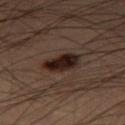notes: imaged on a skin check; not biopsied
anatomic site: the right thigh
acquisition: ~15 mm crop, total-body skin-cancer survey
lesion size: ~4 mm (longest diameter)
subject: male, approximately 55 years of age
tile lighting: cross-polarized illumination